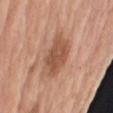follow-up: catalogued during a skin exam; not biopsied
image-analysis metrics: roughly 11 lightness units darker than nearby skin and a lesion-to-skin contrast of about 7.5 (normalized; higher = more distinct); a detector confidence of about 100 out of 100 that the crop contains a lesion
subject: female, aged around 75
diameter: about 5 mm
acquisition: ~15 mm tile from a whole-body skin photo
location: the right upper arm